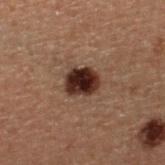Q: Was this lesion biopsied?
A: catalogued during a skin exam; not biopsied